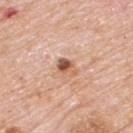This image is a 15 mm lesion crop taken from a total-body photograph. A male patient, aged approximately 80. About 2.5 mm across. Located on the upper back. The total-body-photography lesion software estimated an average lesion color of about L≈57 a*≈23 b*≈32 (CIELAB), roughly 14 lightness units darker than nearby skin, and a lesion-to-skin contrast of about 9 (normalized; higher = more distinct). The analysis additionally found an automated nevus-likeness rating near 85 out of 100 and lesion-presence confidence of about 100/100.